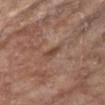site: chest
image:
  source: total-body photography crop
  field_of_view_mm: 15
patient:
  sex: female
  age_approx: 75